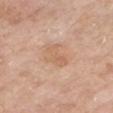notes: catalogued during a skin exam; not biopsied
lighting: white-light illumination
subject: female, in their mid-70s
image source: ~15 mm crop, total-body skin-cancer survey
TBP lesion metrics: a lesion–skin lightness drop of about 6 and a normalized lesion–skin contrast near 5; a border-irregularity index near 3.5/10 and a color-variation rating of about 3/10
location: the chest